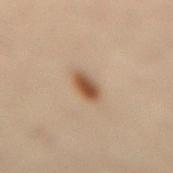The lesion was tiled from a total-body skin photograph and was not biopsied. The patient is a female aged around 30. The lesion's longest dimension is about 2.5 mm. A 15 mm close-up tile from a total-body photography series done for melanoma screening. On the lower back.